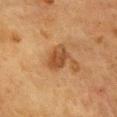Imaged during a routine full-body skin examination; the lesion was not biopsied and no histopathology is available. This is a cross-polarized tile. A female patient approximately 55 years of age. A close-up tile cropped from a whole-body skin photograph, about 15 mm across. Located on the chest. Automated tile analysis of the lesion measured a nevus-likeness score of about 70/100. The recorded lesion diameter is about 3 mm.